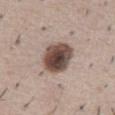{
  "biopsy_status": "not biopsied; imaged during a skin examination",
  "site": "abdomen",
  "lesion_size": {
    "long_diameter_mm_approx": 4.5
  },
  "patient": {
    "sex": "male",
    "age_approx": 50
  },
  "image": {
    "source": "total-body photography crop",
    "field_of_view_mm": 15
  },
  "lighting": "white-light"
}